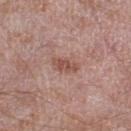Impression:
Imaged during a routine full-body skin examination; the lesion was not biopsied and no histopathology is available.
Acquisition and patient details:
A male patient in their mid-60s. A lesion tile, about 15 mm wide, cut from a 3D total-body photograph. Captured under white-light illumination. Longest diameter approximately 3 mm. The lesion is on the left lower leg.